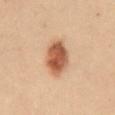biopsy status — catalogued during a skin exam; not biopsied | size — ≈5.5 mm | illumination — cross-polarized illumination | acquisition — 15 mm crop, total-body photography | anatomic site — the mid back | subject — female, aged 28 to 32 | automated lesion analysis — a mean CIELAB color near L≈45 a*≈19 b*≈28, a lesion–skin lightness drop of about 13, and a normalized border contrast of about 10; a border-irregularity index near 2/10, a color-variation rating of about 4/10, and a peripheral color-asymmetry measure near 1.5.